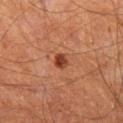workup — no biopsy performed (imaged during a skin exam) | diameter — ~2 mm (longest diameter) | imaging modality — total-body-photography crop, ~15 mm field of view | anatomic site — the right thigh | lighting — cross-polarized illumination | image-analysis metrics — border irregularity of about 2 on a 0–10 scale and peripheral color asymmetry of about 1 | patient — male, aged 63 to 67.